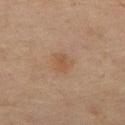biopsy status=total-body-photography surveillance lesion; no biopsy | tile lighting=cross-polarized | subject=female, approximately 70 years of age | image=total-body-photography crop, ~15 mm field of view | location=the left thigh | lesion diameter=about 3 mm.